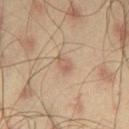Recorded during total-body skin imaging; not selected for excision or biopsy. The subject is a male aged approximately 45. From the left thigh. This image is a 15 mm lesion crop taken from a total-body photograph. Captured under cross-polarized illumination.